The lesion is on the head or neck · a male subject aged 48 to 52 · a close-up tile cropped from a whole-body skin photograph, about 15 mm across:
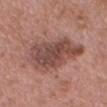{
  "diagnosis": {
    "histopathology": "melanoma in situ",
    "malignancy": "malignant",
    "taxonomic_path": [
      "Malignant",
      "Malignant melanocytic proliferations (Melanoma)",
      "Melanoma in situ"
    ]
  }
}